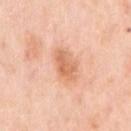{"biopsy_status": "not biopsied; imaged during a skin examination", "image": {"source": "total-body photography crop", "field_of_view_mm": 15}, "site": "right upper arm", "automated_metrics": {"eccentricity": 0.75, "shape_asymmetry": 0.2, "cielab_L": 68, "cielab_a": 25, "cielab_b": 37, "vs_skin_darker_L": 10.0, "vs_skin_contrast_norm": 7.0, "nevus_likeness_0_100": 15}, "patient": {"sex": "female", "age_approx": 65}, "lighting": "white-light"}